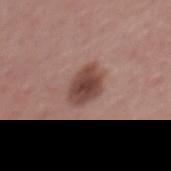The lesion was tiled from a total-body skin photograph and was not biopsied.
A male subject aged 38 to 42.
Located on the mid back.
Captured under white-light illumination.
The total-body-photography lesion software estimated a classifier nevus-likeness of about 80/100 and a lesion-detection confidence of about 100/100.
This image is a 15 mm lesion crop taken from a total-body photograph.